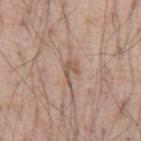Imaged during a routine full-body skin examination; the lesion was not biopsied and no histopathology is available.
A male subject, approximately 55 years of age.
The lesion-visualizer software estimated an area of roughly 3 mm², an eccentricity of roughly 0.8, and a shape-asymmetry score of about 0.55 (0 = symmetric). The analysis additionally found an average lesion color of about L≈55 a*≈17 b*≈27 (CIELAB) and a normalized border contrast of about 6.5.
Located on the abdomen.
A 15 mm crop from a total-body photograph taken for skin-cancer surveillance.
Captured under white-light illumination.